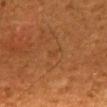Imaged during a routine full-body skin examination; the lesion was not biopsied and no histopathology is available. Captured under cross-polarized illumination. About 1 mm across. The subject is a male roughly 45 years of age. The lesion is located on the mid back. Cropped from a whole-body photographic skin survey; the tile spans about 15 mm.